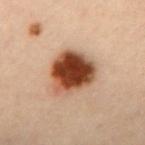follow-up: imaged on a skin check; not biopsied.